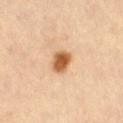lighting = cross-polarized | size = about 3 mm | acquisition = 15 mm crop, total-body photography | body site = the abdomen.